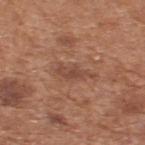Impression:
Part of a total-body skin-imaging series; this lesion was reviewed on a skin check and was not flagged for biopsy.
Background:
Cropped from a whole-body photographic skin survey; the tile spans about 15 mm. The lesion is on the upper back. Imaged with white-light lighting. A male subject, about 65 years old.